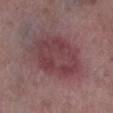workup — no biopsy performed (imaged during a skin exam)
image-analysis metrics — an eccentricity of roughly 0.65 and a symmetry-axis asymmetry near 0.15; a mean CIELAB color near L≈41 a*≈24 b*≈16, roughly 8 lightness units darker than nearby skin, and a lesion-to-skin contrast of about 7 (normalized; higher = more distinct)
site — the right lower leg
subject — male, aged 68 to 72
acquisition — ~15 mm crop, total-body skin-cancer survey
illumination — white-light